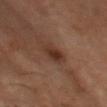No biopsy was performed on this lesion — it was imaged during a full skin examination and was not determined to be concerning.
A male patient aged around 50.
Located on the head or neck.
The total-body-photography lesion software estimated an average lesion color of about L≈27 a*≈16 b*≈22 (CIELAB), roughly 8 lightness units darker than nearby skin, and a normalized lesion–skin contrast near 8.5. It also reported a border-irregularity index near 2.5/10, internal color variation of about 3.5 on a 0–10 scale, and a peripheral color-asymmetry measure near 1.5. The software also gave a lesion-detection confidence of about 100/100.
Cropped from a total-body skin-imaging series; the visible field is about 15 mm.